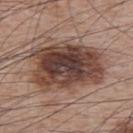Findings:
– biopsy status · no biopsy performed (imaged during a skin exam)
– image · ~15 mm crop, total-body skin-cancer survey
– site · the upper back
– subject · male, aged around 65
– TBP lesion metrics · an area of roughly 36 mm², a shape eccentricity near 0.8, and a symmetry-axis asymmetry near 0.15; a classifier nevus-likeness of about 65/100 and lesion-presence confidence of about 100/100
– tile lighting · white-light
– diameter · about 8.5 mm